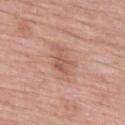Assessment: The lesion was tiled from a total-body skin photograph and was not biopsied. Acquisition and patient details: Captured under white-light illumination. A roughly 15 mm field-of-view crop from a total-body skin photograph. On the left thigh. The patient is a female approximately 70 years of age.